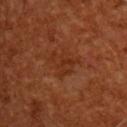<case>
  <biopsy_status>not biopsied; imaged during a skin examination</biopsy_status>
  <lighting>cross-polarized</lighting>
  <patient>
    <sex>male</sex>
    <age_approx>60</age_approx>
  </patient>
  <site>upper back</site>
  <image>
    <source>total-body photography crop</source>
    <field_of_view_mm>15</field_of_view_mm>
  </image>
  <lesion_size>
    <long_diameter_mm_approx>3.5</long_diameter_mm_approx>
  </lesion_size>
</case>